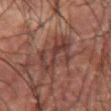Acquisition and patient details:
A male subject, in their mid- to late 50s. A close-up tile cropped from a whole-body skin photograph, about 15 mm across. The lesion is on the right upper arm.